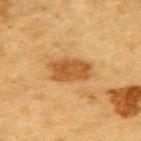Case summary:
• biopsy status: no biopsy performed (imaged during a skin exam)
• lesion size: about 4.5 mm
• subject: male, approximately 85 years of age or older
• body site: the upper back
• automated metrics: an average lesion color of about L≈47 a*≈21 b*≈40 (CIELAB), about 11 CIELAB-L* units darker than the surrounding skin, and a normalized border contrast of about 8.5; border irregularity of about 2 on a 0–10 scale, a color-variation rating of about 2.5/10, and peripheral color asymmetry of about 1; a classifier nevus-likeness of about 90/100 and a detector confidence of about 100 out of 100 that the crop contains a lesion
• acquisition: ~15 mm crop, total-body skin-cancer survey